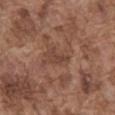Findings:
• workup · imaged on a skin check; not biopsied
• lesion size · ≈3 mm
• image-analysis metrics · a mean CIELAB color near L≈42 a*≈19 b*≈27 and roughly 7 lightness units darker than nearby skin
• subject · male, in their mid-70s
• anatomic site · the abdomen
• acquisition · total-body-photography crop, ~15 mm field of view
• tile lighting · white-light